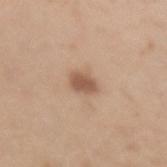subject=female, aged 38–42
lesion diameter=about 2.5 mm
image source=~15 mm tile from a whole-body skin photo
illumination=white-light
location=the left forearm